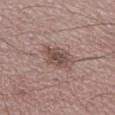Recorded during total-body skin imaging; not selected for excision or biopsy. The tile uses white-light illumination. A male subject, in their 50s. The lesion's longest dimension is about 3 mm. The lesion-visualizer software estimated a lesion area of about 6.5 mm². The software also gave a mean CIELAB color near L≈48 a*≈18 b*≈21, a lesion–skin lightness drop of about 10, and a normalized border contrast of about 7.5. It also reported border irregularity of about 2 on a 0–10 scale, a color-variation rating of about 3/10, and a peripheral color-asymmetry measure near 1. The analysis additionally found a detector confidence of about 100 out of 100 that the crop contains a lesion. Cropped from a total-body skin-imaging series; the visible field is about 15 mm. Located on the left lower leg.